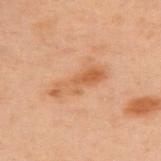Captured during whole-body skin photography for melanoma surveillance; the lesion was not biopsied. From the back. This image is a 15 mm lesion crop taken from a total-body photograph. A female patient, approximately 55 years of age. Automated tile analysis of the lesion measured border irregularity of about 4.5 on a 0–10 scale, a color-variation rating of about 5/10, and radial color variation of about 2.